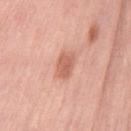| feature | finding |
|---|---|
| workup | imaged on a skin check; not biopsied |
| lesion size | ~3 mm (longest diameter) |
| site | the left thigh |
| acquisition | total-body-photography crop, ~15 mm field of view |
| patient | female, approximately 50 years of age |
| tile lighting | white-light illumination |